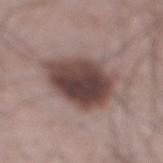Notes:
– follow-up — imaged on a skin check; not biopsied
– illumination — white-light
– site — the back
– image source — 15 mm crop, total-body photography
– lesion diameter — ~6.5 mm (longest diameter)
– subject — male, aged around 65
– automated metrics — an outline eccentricity of about 0.6 (0 = round, 1 = elongated) and two-axis asymmetry of about 0.2; a nevus-likeness score of about 20/100 and lesion-presence confidence of about 100/100